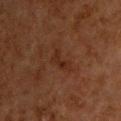follow-up = catalogued during a skin exam; not biopsied | TBP lesion metrics = a lesion area of about 3.5 mm², an eccentricity of roughly 0.9, and a symmetry-axis asymmetry near 0.55; an automated nevus-likeness rating near 0 out of 100 and a lesion-detection confidence of about 100/100 | lighting = cross-polarized | image = total-body-photography crop, ~15 mm field of view | anatomic site = the upper back | subject = male, aged 63–67.